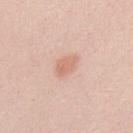No biopsy was performed on this lesion — it was imaged during a full skin examination and was not determined to be concerning.
The lesion-visualizer software estimated a lesion area of about 4.5 mm² and a shape eccentricity near 0.8. And it measured a lesion-detection confidence of about 100/100.
A 15 mm crop from a total-body photograph taken for skin-cancer surveillance.
About 3 mm across.
The subject is a male aged 23 to 27.
This is a white-light tile.
From the upper back.